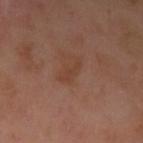No biopsy was performed on this lesion — it was imaged during a full skin examination and was not determined to be concerning.
The total-body-photography lesion software estimated a lesion color around L≈41 a*≈21 b*≈29 in CIELAB, about 5 CIELAB-L* units darker than the surrounding skin, and a normalized lesion–skin contrast near 5.5.
On the left upper arm.
This is a cross-polarized tile.
Cropped from a total-body skin-imaging series; the visible field is about 15 mm.
The subject is a female aged 53 to 57.
Measured at roughly 3.5 mm in maximum diameter.